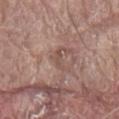This lesion was catalogued during total-body skin photography and was not selected for biopsy. The patient is a male aged 78 to 82. Longest diameter approximately 3 mm. Located on the abdomen. Cropped from a total-body skin-imaging series; the visible field is about 15 mm.